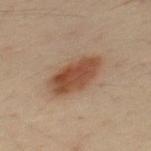Recorded during total-body skin imaging; not selected for excision or biopsy. A 15 mm close-up tile from a total-body photography series done for melanoma screening. A male patient, in their 50s. The lesion is on the back.